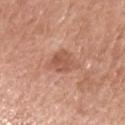This lesion was catalogued during total-body skin photography and was not selected for biopsy.
Approximately 3 mm at its widest.
This is a white-light tile.
Automated tile analysis of the lesion measured a lesion area of about 6.5 mm², an eccentricity of roughly 0.5, and a symmetry-axis asymmetry near 0.35. It also reported a detector confidence of about 100 out of 100 that the crop contains a lesion.
A roughly 15 mm field-of-view crop from a total-body skin photograph.
A female patient in their mid- to late 70s.
From the left upper arm.